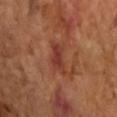Q: Automated lesion metrics?
A: border irregularity of about 4.5 on a 0–10 scale and a color-variation rating of about 2/10; lesion-presence confidence of about 100/100
Q: How was the tile lit?
A: cross-polarized illumination
Q: What is the anatomic site?
A: the arm
Q: Patient demographics?
A: female, aged around 70
Q: What kind of image is this?
A: total-body-photography crop, ~15 mm field of view
Q: What is the lesion's diameter?
A: about 3.5 mm